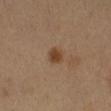follow-up: no biopsy performed (imaged during a skin exam)
subject: male, aged approximately 50
automated metrics: a lesion area of about 4 mm² and a shape-asymmetry score of about 0.1 (0 = symmetric); a border-irregularity rating of about 1/10, internal color variation of about 2 on a 0–10 scale, and a peripheral color-asymmetry measure near 0.5; a lesion-detection confidence of about 100/100
tile lighting: cross-polarized illumination
acquisition: ~15 mm tile from a whole-body skin photo
lesion diameter: about 2.5 mm
body site: the left arm